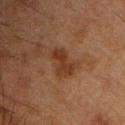follow-up: catalogued during a skin exam; not biopsied
body site: the chest
lighting: cross-polarized
automated lesion analysis: an area of roughly 8 mm², an outline eccentricity of about 0.8 (0 = round, 1 = elongated), and two-axis asymmetry of about 0.25; a border-irregularity index near 3.5/10, a within-lesion color-variation index near 2.5/10, and a peripheral color-asymmetry measure near 1; an automated nevus-likeness rating near 5 out of 100 and a detector confidence of about 100 out of 100 that the crop contains a lesion
lesion size: ~4 mm (longest diameter)
subject: male, aged around 50
imaging modality: 15 mm crop, total-body photography